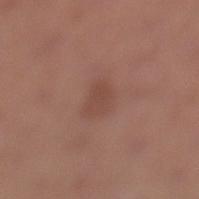Q: What kind of image is this?
A: total-body-photography crop, ~15 mm field of view
Q: Lesion location?
A: the left lower leg
Q: What is the lesion's diameter?
A: about 3 mm
Q: What did automated image analysis measure?
A: a lesion area of about 6 mm² and an outline eccentricity of about 0.6 (0 = round, 1 = elongated); a mean CIELAB color near L≈46 a*≈21 b*≈26, a lesion–skin lightness drop of about 6, and a normalized border contrast of about 5; border irregularity of about 2.5 on a 0–10 scale, a color-variation rating of about 2/10, and radial color variation of about 0.5
Q: Who is the patient?
A: female, aged 48 to 52
Q: What lighting was used for the tile?
A: white-light illumination